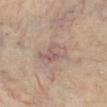notes: total-body-photography surveillance lesion; no biopsy
body site: the right lower leg
subject: female, aged 63 to 67
acquisition: total-body-photography crop, ~15 mm field of view